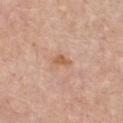workup = catalogued during a skin exam; not biopsied
subject = male, aged 73 to 77
image-analysis metrics = roughly 9 lightness units darker than nearby skin and a normalized border contrast of about 7; a nevus-likeness score of about 0/100 and lesion-presence confidence of about 100/100
body site = the chest
lesion diameter = about 2.5 mm
imaging modality = total-body-photography crop, ~15 mm field of view
tile lighting = white-light illumination